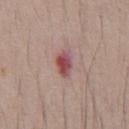Case summary:
– notes: no biopsy performed (imaged during a skin exam)
– subject: male, aged around 40
– lesion diameter: ~3.5 mm (longest diameter)
– image: ~15 mm tile from a whole-body skin photo
– anatomic site: the abdomen